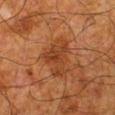biopsy status = no biopsy performed (imaged during a skin exam)
acquisition = ~15 mm tile from a whole-body skin photo
TBP lesion metrics = a mean CIELAB color near L≈31 a*≈21 b*≈30 and a lesion–skin lightness drop of about 7
body site = the left lower leg
diameter = ≈5 mm
patient = male, approximately 80 years of age
illumination = cross-polarized